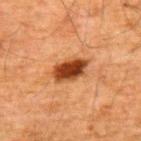image source: ~15 mm crop, total-body skin-cancer survey | site: the back | patient: male, roughly 60 years of age | illumination: cross-polarized | lesion diameter: ≈4.5 mm.